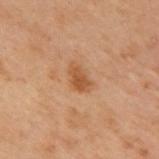| key | value |
|---|---|
| lesion diameter | ~3 mm (longest diameter) |
| image source | ~15 mm tile from a whole-body skin photo |
| tile lighting | cross-polarized |
| location | the upper back |
| subject | male, about 55 years old |
| automated metrics | a border-irregularity index near 2.5/10, a color-variation rating of about 2/10, and radial color variation of about 0.5 |